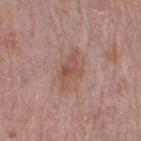Case summary:
* biopsy status: total-body-photography surveillance lesion; no biopsy
* image source: total-body-photography crop, ~15 mm field of view
* body site: the leg
* size: about 5 mm
* automated lesion analysis: a symmetry-axis asymmetry near 0.3; an average lesion color of about L≈52 a*≈21 b*≈26 (CIELAB), about 8 CIELAB-L* units darker than the surrounding skin, and a normalized border contrast of about 6; a border-irregularity rating of about 3.5/10, internal color variation of about 4.5 on a 0–10 scale, and peripheral color asymmetry of about 1.5; an automated nevus-likeness rating near 20 out of 100 and lesion-presence confidence of about 100/100
* patient: female, aged 38–42
* lighting: white-light